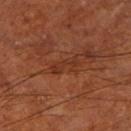follow-up = total-body-photography surveillance lesion; no biopsy | patient = male, aged approximately 65 | illumination = cross-polarized illumination | image source = total-body-photography crop, ~15 mm field of view | diameter = ~3.5 mm (longest diameter) | location = the left lower leg.